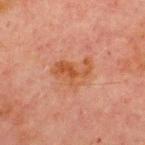Part of a total-body skin-imaging series; this lesion was reviewed on a skin check and was not flagged for biopsy.